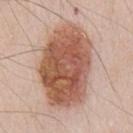| field | value |
|---|---|
| biopsy status | total-body-photography surveillance lesion; no biopsy |
| image | ~15 mm crop, total-body skin-cancer survey |
| subject | male, aged around 45 |
| body site | the front of the torso |